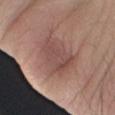notes — imaged on a skin check; not biopsied | illumination — white-light illumination | body site — the right forearm | image source — ~15 mm crop, total-body skin-cancer survey | subject — male, in their mid- to late 30s | size — ≈4 mm.